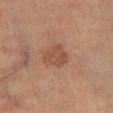biopsy status = total-body-photography surveillance lesion; no biopsy
site = the left lower leg
patient = male, approximately 70 years of age
image = 15 mm crop, total-body photography
lighting = cross-polarized illumination
lesion diameter = about 3 mm